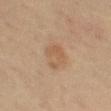Notes:
• follow-up · total-body-photography surveillance lesion; no biopsy
• image · ~15 mm crop, total-body skin-cancer survey
• tile lighting · cross-polarized
• patient · male, aged approximately 65
• automated lesion analysis · an area of roughly 6 mm² and a shape-asymmetry score of about 0.45 (0 = symmetric); a border-irregularity index near 5.5/10 and radial color variation of about 0.5; a detector confidence of about 100 out of 100 that the crop contains a lesion
• site · the leg
• lesion diameter · about 3.5 mm